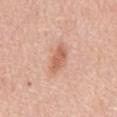Impression: Imaged during a routine full-body skin examination; the lesion was not biopsied and no histopathology is available. Acquisition and patient details: Longest diameter approximately 4 mm. A female patient, approximately 65 years of age. A roughly 15 mm field-of-view crop from a total-body skin photograph. From the mid back. The tile uses white-light illumination. Automated image analysis of the tile measured a lesion color around L≈62 a*≈25 b*≈32 in CIELAB and roughly 11 lightness units darker than nearby skin.